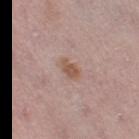follow-up — catalogued during a skin exam; not biopsied
lesion size — ~3 mm (longest diameter)
image source — total-body-photography crop, ~15 mm field of view
subject — female, aged approximately 50
image-analysis metrics — a lesion area of about 4 mm², an eccentricity of roughly 0.85, and a shape-asymmetry score of about 0.3 (0 = symmetric); a border-irregularity index near 3/10, internal color variation of about 1 on a 0–10 scale, and peripheral color asymmetry of about 0.5
site — the right thigh